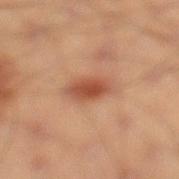| feature | finding |
|---|---|
| notes | catalogued during a skin exam; not biopsied |
| subject | male, approximately 40 years of age |
| image source | 15 mm crop, total-body photography |
| body site | the left lower leg |